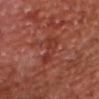The lesion was photographed on a routine skin check and not biopsied; there is no pathology result.
A male patient, aged 63–67.
An algorithmic analysis of the crop reported two-axis asymmetry of about 0.35. It also reported a lesion color around L≈36 a*≈29 b*≈28 in CIELAB, about 7 CIELAB-L* units darker than the surrounding skin, and a normalized border contrast of about 6. It also reported an automated nevus-likeness rating near 0 out of 100.
This is a cross-polarized tile.
The lesion's longest dimension is about 4.5 mm.
Cropped from a whole-body photographic skin survey; the tile spans about 15 mm.